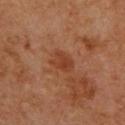Recorded during total-body skin imaging; not selected for excision or biopsy.
The tile uses cross-polarized illumination.
A 15 mm close-up tile from a total-body photography series done for melanoma screening.
A male patient aged 58 to 62.
The recorded lesion diameter is about 3 mm.
The lesion-visualizer software estimated a lesion color around L≈40 a*≈25 b*≈33 in CIELAB, about 7 CIELAB-L* units darker than the surrounding skin, and a lesion-to-skin contrast of about 6.5 (normalized; higher = more distinct). The analysis additionally found a border-irregularity rating of about 3/10, a color-variation rating of about 2/10, and peripheral color asymmetry of about 0.5. It also reported a classifier nevus-likeness of about 0/100 and a lesion-detection confidence of about 100/100.
On the back.